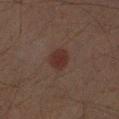{"biopsy_status": "not biopsied; imaged during a skin examination", "patient": {"sex": "male", "age_approx": 45}, "site": "right forearm", "image": {"source": "total-body photography crop", "field_of_view_mm": 15}}